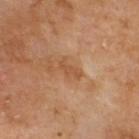{
  "biopsy_status": "not biopsied; imaged during a skin examination",
  "automated_metrics": {
    "area_mm2_approx": 3.0,
    "eccentricity": 0.9,
    "cielab_L": 50,
    "cielab_a": 22,
    "cielab_b": 36,
    "vs_skin_darker_L": 7.0,
    "vs_skin_contrast_norm": 5.5,
    "nevus_likeness_0_100": 0
  },
  "lesion_size": {
    "long_diameter_mm_approx": 3.0
  },
  "image": {
    "source": "total-body photography crop",
    "field_of_view_mm": 15
  },
  "patient": {
    "sex": "male",
    "age_approx": 70
  },
  "lighting": "cross-polarized",
  "site": "upper back"
}